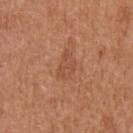This lesion was catalogued during total-body skin photography and was not selected for biopsy. The tile uses white-light illumination. The lesion is on the left upper arm. A female patient, aged 38 to 42. The lesion's longest dimension is about 3.5 mm. A 15 mm close-up tile from a total-body photography series done for melanoma screening.